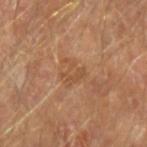| feature | finding |
|---|---|
| notes | no biopsy performed (imaged during a skin exam) |
| automated metrics | an area of roughly 5 mm² and an outline eccentricity of about 0.45 (0 = round, 1 = elongated); a border-irregularity rating of about 6/10, a within-lesion color-variation index near 2/10, and a peripheral color-asymmetry measure near 0.5; a classifier nevus-likeness of about 0/100 |
| image source | ~15 mm tile from a whole-body skin photo |
| lighting | cross-polarized illumination |
| lesion size | about 3 mm |
| site | the arm |
| patient | male, aged 63–67 |